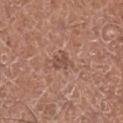Assessment: Imaged during a routine full-body skin examination; the lesion was not biopsied and no histopathology is available. Image and clinical context: This is a white-light tile. A 15 mm close-up tile from a total-body photography series done for melanoma screening. Located on the leg. A female subject approximately 50 years of age. Automated tile analysis of the lesion measured a nevus-likeness score of about 0/100 and lesion-presence confidence of about 100/100. Approximately 2.5 mm at its widest.